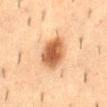Assessment:
Part of a total-body skin-imaging series; this lesion was reviewed on a skin check and was not flagged for biopsy.
Clinical summary:
The recorded lesion diameter is about 4.5 mm. The lesion is on the mid back. A male subject about 55 years old. The tile uses cross-polarized illumination. Automated image analysis of the tile measured an area of roughly 12 mm², an eccentricity of roughly 0.6, and two-axis asymmetry of about 0.1. And it measured an average lesion color of about L≈53 a*≈22 b*≈35 (CIELAB), roughly 15 lightness units darker than nearby skin, and a normalized border contrast of about 10.5. The analysis additionally found a classifier nevus-likeness of about 100/100 and lesion-presence confidence of about 100/100. A 15 mm close-up extracted from a 3D total-body photography capture.